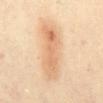Case summary:
– follow-up: catalogued during a skin exam; not biopsied
– lighting: cross-polarized
– subject: female, aged approximately 35
– imaging modality: 15 mm crop, total-body photography
– anatomic site: the chest
– lesion size: ~7.5 mm (longest diameter)
– automated lesion analysis: a classifier nevus-likeness of about 95/100 and a lesion-detection confidence of about 100/100